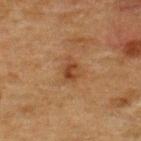A male patient, roughly 50 years of age.
A 15 mm crop from a total-body photograph taken for skin-cancer surveillance.
About 3 mm across.
The lesion is on the upper back.